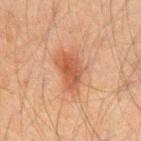Q: Was this lesion biopsied?
A: catalogued during a skin exam; not biopsied
Q: How large is the lesion?
A: ~4.5 mm (longest diameter)
Q: Patient demographics?
A: male, aged 58 to 62
Q: How was this image acquired?
A: 15 mm crop, total-body photography
Q: What is the anatomic site?
A: the chest
Q: Automated lesion metrics?
A: a lesion color around L≈42 a*≈21 b*≈29 in CIELAB, roughly 9 lightness units darker than nearby skin, and a normalized border contrast of about 7.5; border irregularity of about 3 on a 0–10 scale, a within-lesion color-variation index near 2.5/10, and a peripheral color-asymmetry measure near 1; a nevus-likeness score of about 95/100
Q: Illumination type?
A: cross-polarized